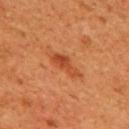Notes:
– lighting — cross-polarized
– image — ~15 mm tile from a whole-body skin photo
– diameter — about 4 mm
– location — the upper back
– patient — male, in their mid- to late 40s